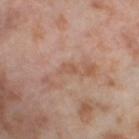• workup · no biopsy performed (imaged during a skin exam)
• anatomic site · the left thigh
• lesion size · ≈2.5 mm
• acquisition · ~15 mm crop, total-body skin-cancer survey
• subject · female, about 55 years old